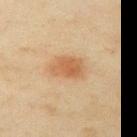<lesion>
<biopsy_status>not biopsied; imaged during a skin examination</biopsy_status>
<lighting>cross-polarized</lighting>
<patient>
  <sex>male</sex>
  <age_approx>45</age_approx>
</patient>
<lesion_size>
  <long_diameter_mm_approx>3.5</long_diameter_mm_approx>
</lesion_size>
<image>
  <source>total-body photography crop</source>
  <field_of_view_mm>15</field_of_view_mm>
</image>
<site>left upper arm</site>
</lesion>